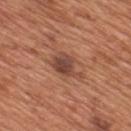Impression:
The lesion was tiled from a total-body skin photograph and was not biopsied.
Background:
A region of skin cropped from a whole-body photographic capture, roughly 15 mm wide. The lesion-visualizer software estimated a footprint of about 7.5 mm², a shape eccentricity near 0.75, and two-axis asymmetry of about 0.45. The analysis additionally found a mean CIELAB color near L≈45 a*≈23 b*≈28 and about 11 CIELAB-L* units darker than the surrounding skin. The software also gave a border-irregularity index near 4.5/10, a color-variation rating of about 4.5/10, and a peripheral color-asymmetry measure near 1.5. A male subject in their mid- to late 60s. From the mid back. This is a white-light tile.